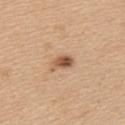Findings:
- location — the upper back
- lesion size — about 3 mm
- acquisition — ~15 mm crop, total-body skin-cancer survey
- subject — female, in their mid- to late 40s